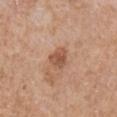  biopsy_status: not biopsied; imaged during a skin examination
  site: right upper arm
  patient:
    sex: male
    age_approx: 65
  lesion_size:
    long_diameter_mm_approx: 2.5
  lighting: white-light
  image:
    source: total-body photography crop
    field_of_view_mm: 15